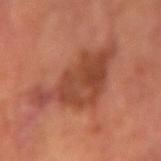biopsy status — imaged on a skin check; not biopsied | image source — ~15 mm crop, total-body skin-cancer survey | patient — male, about 65 years old | location — the left forearm | size — about 9.5 mm.